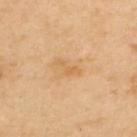Impression: Imaged during a routine full-body skin examination; the lesion was not biopsied and no histopathology is available. Background: The lesion-visualizer software estimated about 7 CIELAB-L* units darker than the surrounding skin. And it measured a border-irregularity rating of about 7/10, a color-variation rating of about 0/10, and a peripheral color-asymmetry measure near 0. Located on the upper back. About 3 mm across. Cropped from a total-body skin-imaging series; the visible field is about 15 mm. A male patient aged 53 to 57.